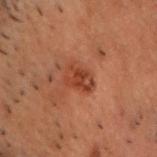* notes · imaged on a skin check; not biopsied
* lighting · cross-polarized
* subject · male, aged 48 to 52
* body site · the head or neck
* image · ~15 mm tile from a whole-body skin photo